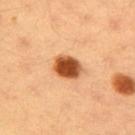No biopsy was performed on this lesion — it was imaged during a full skin examination and was not determined to be concerning. The recorded lesion diameter is about 3.5 mm. A female subject approximately 35 years of age. A 15 mm close-up tile from a total-body photography series done for melanoma screening. From the right upper arm. Imaged with cross-polarized lighting.